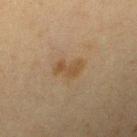The tile uses cross-polarized illumination.
A female patient in their 40s.
This image is a 15 mm lesion crop taken from a total-body photograph.
The lesion-visualizer software estimated a lesion area of about 5 mm² and an eccentricity of roughly 0.8. And it measured a lesion color around L≈40 a*≈13 b*≈29 in CIELAB, about 6 CIELAB-L* units darker than the surrounding skin, and a normalized border contrast of about 6.5. And it measured an automated nevus-likeness rating near 10 out of 100 and a detector confidence of about 100 out of 100 that the crop contains a lesion.
About 3.5 mm across.
Located on the right upper arm.